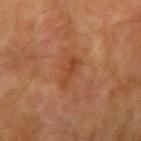The lesion was tiled from a total-body skin photograph and was not biopsied.
The subject is a male aged approximately 75.
Automated image analysis of the tile measured a lesion area of about 3.5 mm², a shape eccentricity near 0.95, and a symmetry-axis asymmetry near 0.5. The software also gave a classifier nevus-likeness of about 5/100.
Measured at roughly 3.5 mm in maximum diameter.
On the right upper arm.
This image is a 15 mm lesion crop taken from a total-body photograph.